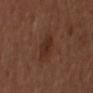Part of a total-body skin-imaging series; this lesion was reviewed on a skin check and was not flagged for biopsy.